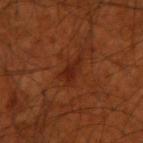Q: Was a biopsy performed?
A: imaged on a skin check; not biopsied
Q: How was this image acquired?
A: total-body-photography crop, ~15 mm field of view
Q: Illumination type?
A: cross-polarized
Q: Who is the patient?
A: male, about 70 years old
Q: What is the anatomic site?
A: the arm
Q: What did automated image analysis measure?
A: an average lesion color of about L≈25 a*≈25 b*≈30 (CIELAB) and a lesion-to-skin contrast of about 7 (normalized; higher = more distinct)
Q: Lesion size?
A: about 3.5 mm